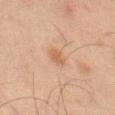Q: Is there a histopathology result?
A: imaged on a skin check; not biopsied
Q: How was the tile lit?
A: cross-polarized illumination
Q: What is the imaging modality?
A: 15 mm crop, total-body photography
Q: Where on the body is the lesion?
A: the left thigh
Q: What are the patient's age and sex?
A: male, in their mid-40s
Q: What did automated image analysis measure?
A: a shape eccentricity near 0.8; peripheral color asymmetry of about 0